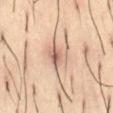workup: imaged on a skin check; not biopsied | body site: the abdomen | lesion diameter: ~3.5 mm (longest diameter) | patient: male, aged approximately 55 | image source: ~15 mm tile from a whole-body skin photo | lighting: cross-polarized.